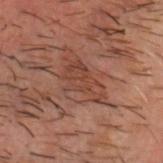Imaged during a routine full-body skin examination; the lesion was not biopsied and no histopathology is available.
A male subject, aged 48 to 52.
The lesion is on the head or neck.
A lesion tile, about 15 mm wide, cut from a 3D total-body photograph.
The tile uses cross-polarized illumination.